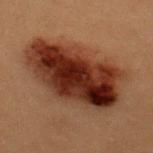  lesion_size:
    long_diameter_mm_approx: 11.0
  lighting: cross-polarized
  patient:
    sex: female
    age_approx: 20
  image:
    source: total-body photography crop
    field_of_view_mm: 15
  site: upper back